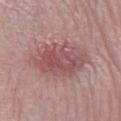Imaged during a routine full-body skin examination; the lesion was not biopsied and no histopathology is available.
A region of skin cropped from a whole-body photographic capture, roughly 15 mm wide.
Imaged with white-light lighting.
The lesion-visualizer software estimated a lesion area of about 22 mm² and an outline eccentricity of about 0.65 (0 = round, 1 = elongated). The software also gave a border-irregularity rating of about 2.5/10, internal color variation of about 4.5 on a 0–10 scale, and radial color variation of about 1.5.
Located on the left lower leg.
A male subject about 40 years old.
About 6.5 mm across.